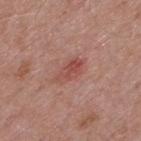Assessment:
Imaged during a routine full-body skin examination; the lesion was not biopsied and no histopathology is available.
Image and clinical context:
A male patient aged around 70. From the upper back. A 15 mm crop from a total-body photograph taken for skin-cancer surveillance.